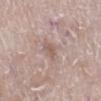The lesion was tiled from a total-body skin photograph and was not biopsied.
About 2.5 mm across.
Imaged with white-light lighting.
A 15 mm close-up extracted from a 3D total-body photography capture.
From the left lower leg.
A male patient, aged approximately 80.
Automated tile analysis of the lesion measured a mean CIELAB color near L≈58 a*≈16 b*≈23, about 7 CIELAB-L* units darker than the surrounding skin, and a normalized border contrast of about 5.5.